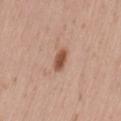Case summary:
• image source — 15 mm crop, total-body photography
• anatomic site — the lower back
• automated metrics — roughly 13 lightness units darker than nearby skin and a normalized border contrast of about 9.5
• subject — female, roughly 30 years of age
• lesion size — about 3 mm
• tile lighting — white-light illumination